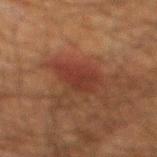On the mid back. A male subject about 60 years old. Cropped from a whole-body photographic skin survey; the tile spans about 15 mm.